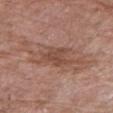workup — catalogued during a skin exam; not biopsied
patient — female, about 70 years old
site — the right upper arm
image — 15 mm crop, total-body photography